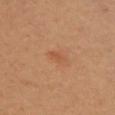No biopsy was performed on this lesion — it was imaged during a full skin examination and was not determined to be concerning. This is a cross-polarized tile. The subject is a female aged approximately 40. Automated tile analysis of the lesion measured a lesion area of about 3 mm², a shape eccentricity near 0.85, and a symmetry-axis asymmetry near 0.3. The software also gave an automated nevus-likeness rating near 5 out of 100. Approximately 2.5 mm at its widest. Cropped from a whole-body photographic skin survey; the tile spans about 15 mm. The lesion is located on the chest.